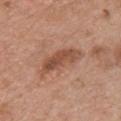Assessment: Part of a total-body skin-imaging series; this lesion was reviewed on a skin check and was not flagged for biopsy. Acquisition and patient details: Located on the chest. Cropped from a whole-body photographic skin survey; the tile spans about 15 mm. A male patient in their 60s.